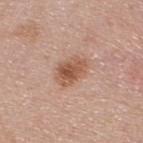Case summary:
- workup — catalogued during a skin exam; not biopsied
- patient — male, aged 43–47
- location — the upper back
- acquisition — ~15 mm tile from a whole-body skin photo